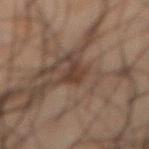{
  "site": "abdomen",
  "automated_metrics": {
    "area_mm2_approx": 3.5,
    "eccentricity": 0.85,
    "shape_asymmetry": 0.5,
    "vs_skin_darker_L": 7.0,
    "vs_skin_contrast_norm": 7.5,
    "border_irregularity_0_10": 5.0,
    "color_variation_0_10": 1.5,
    "peripheral_color_asymmetry": 0.5
  },
  "lesion_size": {
    "long_diameter_mm_approx": 3.5
  },
  "lighting": "cross-polarized",
  "image": {
    "source": "total-body photography crop",
    "field_of_view_mm": 15
  },
  "patient": {
    "sex": "male",
    "age_approx": 50
  }
}